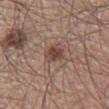notes: no biopsy performed (imaged during a skin exam)
body site: the right lower leg
image: 15 mm crop, total-body photography
automated lesion analysis: a footprint of about 4.5 mm², an outline eccentricity of about 0.55 (0 = round, 1 = elongated), and two-axis asymmetry of about 0.25
subject: male, about 60 years old